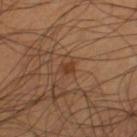The lesion was tiled from a total-body skin photograph and was not biopsied. A 15 mm close-up extracted from a 3D total-body photography capture. The recorded lesion diameter is about 1.5 mm. A male subject approximately 60 years of age. Located on the left thigh.